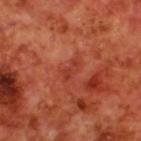Impression: This lesion was catalogued during total-body skin photography and was not selected for biopsy. Image and clinical context: Cropped from a total-body skin-imaging series; the visible field is about 15 mm. The subject is a male aged around 70. The lesion is on the upper back.